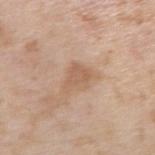Assessment: Imaged during a routine full-body skin examination; the lesion was not biopsied and no histopathology is available. Clinical summary: The lesion is located on the right upper arm. A roughly 15 mm field-of-view crop from a total-body skin photograph. This is a white-light tile. The patient is a male aged 43–47. Longest diameter approximately 3.5 mm. Automated tile analysis of the lesion measured an area of roughly 6.5 mm², an outline eccentricity of about 0.65 (0 = round, 1 = elongated), and two-axis asymmetry of about 0.35. The analysis additionally found an average lesion color of about L≈60 a*≈18 b*≈32 (CIELAB), about 8 CIELAB-L* units darker than the surrounding skin, and a normalized border contrast of about 5.5. It also reported a nevus-likeness score of about 0/100 and a lesion-detection confidence of about 100/100.